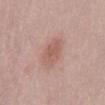This lesion was catalogued during total-body skin photography and was not selected for biopsy. A female subject aged approximately 30. Located on the front of the torso. This image is a 15 mm lesion crop taken from a total-body photograph. Longest diameter approximately 3.5 mm. This is a white-light tile.